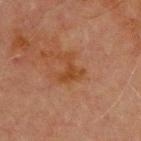Imaged during a routine full-body skin examination; the lesion was not biopsied and no histopathology is available.
A roughly 15 mm field-of-view crop from a total-body skin photograph.
A male subject, aged approximately 70.
Located on the chest.